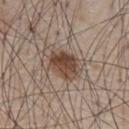The lesion was photographed on a routine skin check and not biopsied; there is no pathology result. On the chest. Captured under white-light illumination. A male patient, aged around 80. Measured at roughly 4 mm in maximum diameter. A 15 mm crop from a total-body photograph taken for skin-cancer surveillance.